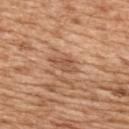This lesion was catalogued during total-body skin photography and was not selected for biopsy. The lesion's longest dimension is about 4 mm. A close-up tile cropped from a whole-body skin photograph, about 15 mm across. Captured under white-light illumination. A female patient, in their mid-50s. On the upper back. An algorithmic analysis of the crop reported about 9 CIELAB-L* units darker than the surrounding skin. And it measured a classifier nevus-likeness of about 0/100 and lesion-presence confidence of about 100/100.